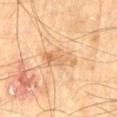Impression:
Captured during whole-body skin photography for melanoma surveillance; the lesion was not biopsied.
Context:
Automated tile analysis of the lesion measured an automated nevus-likeness rating near 0 out of 100 and a lesion-detection confidence of about 100/100. Longest diameter approximately 4 mm. This image is a 15 mm lesion crop taken from a total-body photograph. The subject is a male about 70 years old. The lesion is located on the left thigh. This is a cross-polarized tile.